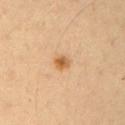notes = no biopsy performed (imaged during a skin exam) | patient = male, roughly 55 years of age | imaging modality = ~15 mm crop, total-body skin-cancer survey | location = the right upper arm.